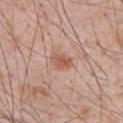<tbp_lesion>
<biopsy_status>not biopsied; imaged during a skin examination</biopsy_status>
<site>abdomen</site>
<patient>
  <sex>male</sex>
  <age_approx>55</age_approx>
</patient>
<image>
  <source>total-body photography crop</source>
  <field_of_view_mm>15</field_of_view_mm>
</image>
<lesion_size>
  <long_diameter_mm_approx>2.5</long_diameter_mm_approx>
</lesion_size>
<lighting>white-light</lighting>
</tbp_lesion>